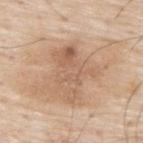| field | value |
|---|---|
| notes | imaged on a skin check; not biopsied |
| image source | ~15 mm tile from a whole-body skin photo |
| subject | male, aged 78–82 |
| body site | the upper back |